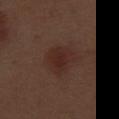notes: no biopsy performed (imaged during a skin exam) | site: the right thigh | image source: ~15 mm crop, total-body skin-cancer survey | patient: male, approximately 70 years of age.